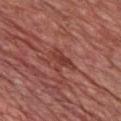Case summary:
- notes: total-body-photography surveillance lesion; no biopsy
- image: ~15 mm tile from a whole-body skin photo
- illumination: white-light
- patient: male, aged 58–62
- body site: the chest